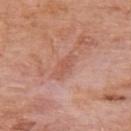The lesion was tiled from a total-body skin photograph and was not biopsied. The lesion is located on the upper back. The subject is a male aged approximately 75. A lesion tile, about 15 mm wide, cut from a 3D total-body photograph.